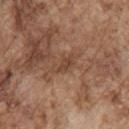Notes:
• workup: catalogued during a skin exam; not biopsied
• tile lighting: white-light
• diameter: ~3.5 mm (longest diameter)
• patient: male, roughly 75 years of age
• site: the right upper arm
• image: ~15 mm tile from a whole-body skin photo